A male patient, aged approximately 65; a region of skin cropped from a whole-body photographic capture, roughly 15 mm wide; located on the mid back; the tile uses white-light illumination: 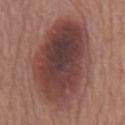Q: What is the histopathologic diagnosis?
A: a melanoma in situ — a malignant lesion